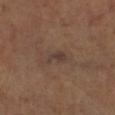<tbp_lesion>
  <biopsy_status>not biopsied; imaged during a skin examination</biopsy_status>
  <lighting>cross-polarized</lighting>
  <patient>
    <sex>female</sex>
    <age_approx>70</age_approx>
  </patient>
  <automated_metrics>
    <cielab_L>38</cielab_L>
    <cielab_a>15</cielab_a>
    <cielab_b>21</cielab_b>
    <vs_skin_darker_L>6.0</vs_skin_darker_L>
    <vs_skin_contrast_norm>7.0</vs_skin_contrast_norm>
    <color_variation_0_10>1.5</color_variation_0_10>
  </automated_metrics>
  <site>left lower leg</site>
  <image>
    <source>total-body photography crop</source>
    <field_of_view_mm>15</field_of_view_mm>
  </image>
  <lesion_size>
    <long_diameter_mm_approx>3.0</long_diameter_mm_approx>
  </lesion_size>
</tbp_lesion>